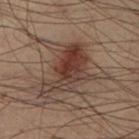Captured during whole-body skin photography for melanoma surveillance; the lesion was not biopsied. A close-up tile cropped from a whole-body skin photograph, about 15 mm across. A male patient, approximately 55 years of age. An algorithmic analysis of the crop reported an outline eccentricity of about 0.8 (0 = round, 1 = elongated) and a symmetry-axis asymmetry near 0.4. And it measured an average lesion color of about L≈30 a*≈15 b*≈20 (CIELAB) and a lesion-to-skin contrast of about 8.5 (normalized; higher = more distinct). And it measured a border-irregularity index near 5.5/10 and internal color variation of about 6.5 on a 0–10 scale. And it measured lesion-presence confidence of about 100/100. On the left lower leg.